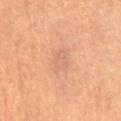biopsy_status: not biopsied; imaged during a skin examination
site: mid back
lighting: cross-polarized
automated_metrics:
  area_mm2_approx: 5.5
  eccentricity: 0.7
  vs_skin_darker_L: 5.0
  vs_skin_contrast_norm: 4.5
  color_variation_0_10: 1.5
  peripheral_color_asymmetry: 0.5
  nevus_likeness_0_100: 0
  lesion_detection_confidence_0_100: 100
patient:
  sex: female
  age_approx: 70
lesion_size:
  long_diameter_mm_approx: 3.0
image:
  source: total-body photography crop
  field_of_view_mm: 15